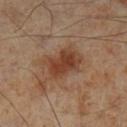Clinical impression: Imaged during a routine full-body skin examination; the lesion was not biopsied and no histopathology is available. Acquisition and patient details: On the left lower leg. A region of skin cropped from a whole-body photographic capture, roughly 15 mm wide. About 5 mm across. The total-body-photography lesion software estimated an average lesion color of about L≈40 a*≈20 b*≈29 (CIELAB), roughly 9 lightness units darker than nearby skin, and a lesion-to-skin contrast of about 8 (normalized; higher = more distinct). The software also gave a border-irregularity index near 2.5/10 and a color-variation rating of about 4/10. The patient is a male aged 68–72. Imaged with cross-polarized lighting.